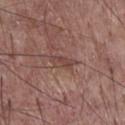imaging modality = ~15 mm tile from a whole-body skin photo; lesion size = about 3.5 mm; location = the chest; tile lighting = white-light illumination; subject = male, roughly 60 years of age.